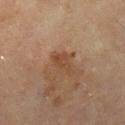automated metrics: border irregularity of about 3.5 on a 0–10 scale and a color-variation rating of about 1.5/10; a nevus-likeness score of about 0/100 and lesion-presence confidence of about 100/100 | patient: female, aged approximately 55 | site: the right lower leg | size: about 3 mm | illumination: cross-polarized illumination | image source: 15 mm crop, total-body photography.